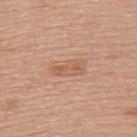Part of a total-body skin-imaging series; this lesion was reviewed on a skin check and was not flagged for biopsy.